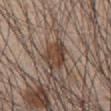Q: Who is the patient?
A: male, in their mid-60s
Q: What is the anatomic site?
A: the abdomen
Q: What kind of image is this?
A: ~15 mm tile from a whole-body skin photo
Q: What lighting was used for the tile?
A: white-light